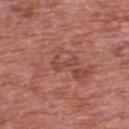| feature | finding |
|---|---|
| workup | imaged on a skin check; not biopsied |
| image | 15 mm crop, total-body photography |
| lighting | white-light |
| diameter | about 3 mm |
| body site | the upper back |
| subject | male, aged approximately 70 |